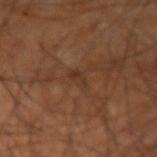| feature | finding |
|---|---|
| biopsy status | imaged on a skin check; not biopsied |
| acquisition | ~15 mm crop, total-body skin-cancer survey |
| location | the left arm |
| illumination | cross-polarized illumination |
| patient | male, roughly 60 years of age |
| automated lesion analysis | two-axis asymmetry of about 0.35; a mean CIELAB color near L≈31 a*≈18 b*≈27, roughly 5 lightness units darker than nearby skin, and a normalized lesion–skin contrast near 5.5; internal color variation of about 0 on a 0–10 scale and radial color variation of about 0 |